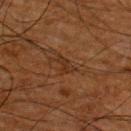<tbp_lesion>
<biopsy_status>not biopsied; imaged during a skin examination</biopsy_status>
<automated_metrics>
  <border_irregularity_0_10>5.5</border_irregularity_0_10>
  <color_variation_0_10>1.5</color_variation_0_10>
</automated_metrics>
<patient>
  <sex>male</sex>
  <age_approx>65</age_approx>
</patient>
<lesion_size>
  <long_diameter_mm_approx>3.0</long_diameter_mm_approx>
</lesion_size>
<site>upper back</site>
<lighting>cross-polarized</lighting>
<image>
  <source>total-body photography crop</source>
  <field_of_view_mm>15</field_of_view_mm>
</image>
</tbp_lesion>